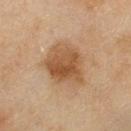The lesion was photographed on a routine skin check and not biopsied; there is no pathology result. Cropped from a total-body skin-imaging series; the visible field is about 15 mm. A female subject, aged 58–62. Located on the left thigh. Measured at roughly 6 mm in maximum diameter.